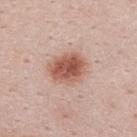The lesion was tiled from a total-body skin photograph and was not biopsied.
A region of skin cropped from a whole-body photographic capture, roughly 15 mm wide.
The lesion is on the upper back.
A male subject aged 23 to 27.